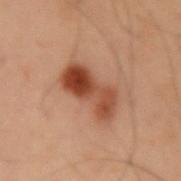Imaged during a routine full-body skin examination; the lesion was not biopsied and no histopathology is available. Cropped from a total-body skin-imaging series; the visible field is about 15 mm. Longest diameter approximately 6.5 mm. From the left upper arm. The patient is a male aged 53–57. Imaged with cross-polarized lighting.